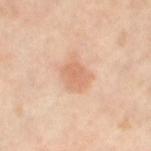From the leg. The subject is a female roughly 50 years of age. A 15 mm crop from a total-body photograph taken for skin-cancer surveillance. Approximately 3.5 mm at its widest.